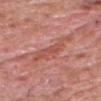{"biopsy_status": "not biopsied; imaged during a skin examination", "lesion_size": {"long_diameter_mm_approx": 6.0}, "site": "head or neck", "patient": {"sex": "male", "age_approx": 60}, "automated_metrics": {"area_mm2_approx": 10.0, "eccentricity": 0.9, "shape_asymmetry": 0.4, "cielab_L": 53, "cielab_a": 28, "cielab_b": 28, "vs_skin_darker_L": 7.0, "vs_skin_contrast_norm": 5.5}, "image": {"source": "total-body photography crop", "field_of_view_mm": 15}}